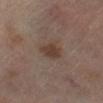{"biopsy_status": "not biopsied; imaged during a skin examination", "site": "right lower leg", "patient": {"sex": "male", "age_approx": 70}, "image": {"source": "total-body photography crop", "field_of_view_mm": 15}, "lighting": "cross-polarized", "automated_metrics": {"cielab_L": 36, "cielab_a": 16, "cielab_b": 23, "vs_skin_darker_L": 8.0, "vs_skin_contrast_norm": 8.0, "nevus_likeness_0_100": 70, "lesion_detection_confidence_0_100": 100}}